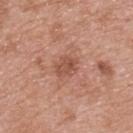Findings:
- patient: female, aged 58–62
- body site: the upper back
- diameter: ~2.5 mm (longest diameter)
- lighting: white-light
- image source: ~15 mm crop, total-body skin-cancer survey